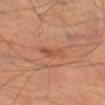Clinical impression:
This lesion was catalogued during total-body skin photography and was not selected for biopsy.
Context:
Cropped from a whole-body photographic skin survey; the tile spans about 15 mm. The patient is a male aged 63 to 67. The lesion is on the right thigh.